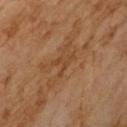| field | value |
|---|---|
| biopsy status | no biopsy performed (imaged during a skin exam) |
| subject | male, roughly 65 years of age |
| imaging modality | total-body-photography crop, ~15 mm field of view |
| size | about 3 mm |